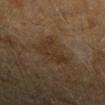Part of a total-body skin-imaging series; this lesion was reviewed on a skin check and was not flagged for biopsy. A 15 mm crop from a total-body photograph taken for skin-cancer surveillance. Located on the right forearm. The patient is a female aged 58–62.